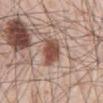• follow-up: no biopsy performed (imaged during a skin exam)
• anatomic site: the abdomen
• subject: male, aged 53–57
• image source: total-body-photography crop, ~15 mm field of view
• size: ~3.5 mm (longest diameter)
• tile lighting: white-light illumination